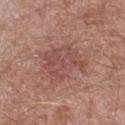The lesion was photographed on a routine skin check and not biopsied; there is no pathology result.
The lesion is located on the right lower leg.
A male subject, aged 58 to 62.
Measured at roughly 6 mm in maximum diameter.
A close-up tile cropped from a whole-body skin photograph, about 15 mm across.